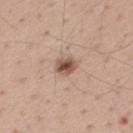Clinical impression:
This lesion was catalogued during total-body skin photography and was not selected for biopsy.
Context:
A lesion tile, about 15 mm wide, cut from a 3D total-body photograph. The tile uses white-light illumination. The subject is a male aged approximately 40. From the back. About 2.5 mm across.